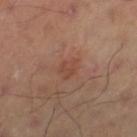workup=no biopsy performed (imaged during a skin exam)
image source=~15 mm tile from a whole-body skin photo
lesion size=about 3 mm
anatomic site=the left thigh